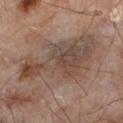| key | value |
|---|---|
| notes | total-body-photography surveillance lesion; no biopsy |
| size | about 9.5 mm |
| automated metrics | an average lesion color of about L≈44 a*≈14 b*≈23 (CIELAB) and a lesion-to-skin contrast of about 6.5 (normalized; higher = more distinct); a classifier nevus-likeness of about 0/100 and lesion-presence confidence of about 85/100 |
| imaging modality | 15 mm crop, total-body photography |
| subject | male, about 65 years old |
| tile lighting | cross-polarized |
| location | the left lower leg |